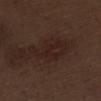- follow-up — imaged on a skin check; not biopsied
- subject — male, aged 68–72
- size — ~5 mm (longest diameter)
- imaging modality — total-body-photography crop, ~15 mm field of view
- location — the left thigh